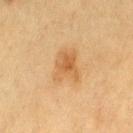| key | value |
|---|---|
| biopsy status | imaged on a skin check; not biopsied |
| lesion diameter | ~3.5 mm (longest diameter) |
| anatomic site | the left thigh |
| patient | female, aged 53–57 |
| image | total-body-photography crop, ~15 mm field of view |